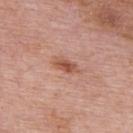Q: Is there a histopathology result?
A: no biopsy performed (imaged during a skin exam)
Q: How was the tile lit?
A: white-light illumination
Q: How was this image acquired?
A: total-body-photography crop, ~15 mm field of view
Q: How large is the lesion?
A: ≈3 mm
Q: Where on the body is the lesion?
A: the upper back
Q: Automated lesion metrics?
A: a footprint of about 3.5 mm², an outline eccentricity of about 0.9 (0 = round, 1 = elongated), and a shape-asymmetry score of about 0.35 (0 = symmetric); a mean CIELAB color near L≈53 a*≈25 b*≈31 and about 11 CIELAB-L* units darker than the surrounding skin; lesion-presence confidence of about 100/100
Q: What are the patient's age and sex?
A: male, aged 73 to 77